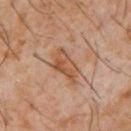Assessment: No biopsy was performed on this lesion — it was imaged during a full skin examination and was not determined to be concerning. Clinical summary: The lesion is located on the front of the torso. About 4.5 mm across. A male subject, aged approximately 60. This image is a 15 mm lesion crop taken from a total-body photograph.